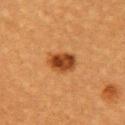Assessment:
Imaged during a routine full-body skin examination; the lesion was not biopsied and no histopathology is available.
Image and clinical context:
This image is a 15 mm lesion crop taken from a total-body photograph. Captured under cross-polarized illumination. Longest diameter approximately 3.5 mm. The subject is a female roughly 55 years of age. The total-body-photography lesion software estimated an eccentricity of roughly 0.7. It also reported a classifier nevus-likeness of about 100/100 and a detector confidence of about 100 out of 100 that the crop contains a lesion. The lesion is located on the chest.